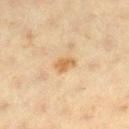Imaged during a routine full-body skin examination; the lesion was not biopsied and no histopathology is available.
A region of skin cropped from a whole-body photographic capture, roughly 15 mm wide.
This is a cross-polarized tile.
Located on the leg.
Approximately 3 mm at its widest.
A female subject aged 38–42.